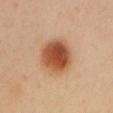Impression:
Imaged during a routine full-body skin examination; the lesion was not biopsied and no histopathology is available.
Acquisition and patient details:
A female subject, roughly 40 years of age. The tile uses cross-polarized illumination. A close-up tile cropped from a whole-body skin photograph, about 15 mm across. Located on the chest.